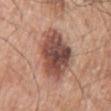A male patient about 70 years old. Longest diameter approximately 6.5 mm. Automated image analysis of the tile measured an eccentricity of roughly 0.7 and a symmetry-axis asymmetry near 0.15. The software also gave a border-irregularity index near 2.5/10, a color-variation rating of about 7.5/10, and peripheral color asymmetry of about 2. The software also gave an automated nevus-likeness rating near 30 out of 100 and a detector confidence of about 100 out of 100 that the crop contains a lesion. From the left arm. Cropped from a whole-body photographic skin survey; the tile spans about 15 mm.